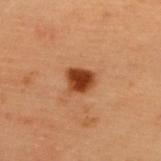Notes:
* workup: total-body-photography surveillance lesion; no biopsy
* subject: female, approximately 40 years of age
* tile lighting: cross-polarized
* body site: the back
* automated lesion analysis: a normalized lesion–skin contrast near 12; a classifier nevus-likeness of about 100/100 and a detector confidence of about 100 out of 100 that the crop contains a lesion
* image source: ~15 mm crop, total-body skin-cancer survey
* size: about 3 mm